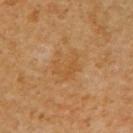{
  "biopsy_status": "not biopsied; imaged during a skin examination",
  "site": "chest",
  "patient": {
    "sex": "female"
  },
  "image": {
    "source": "total-body photography crop",
    "field_of_view_mm": 15
  }
}